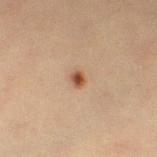biopsy status = catalogued during a skin exam; not biopsied | imaging modality = 15 mm crop, total-body photography | automated lesion analysis = an eccentricity of roughly 0.45 and two-axis asymmetry of about 0.25 | anatomic site = the left thigh | lesion diameter = ≈2 mm | subject = female, aged approximately 40.